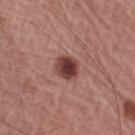Case summary:
* notes · total-body-photography surveillance lesion; no biopsy
* illumination · white-light illumination
* anatomic site · the left upper arm
* TBP lesion metrics · an area of roughly 6.5 mm², an eccentricity of roughly 0.3, and two-axis asymmetry of about 0.15; a mean CIELAB color near L≈41 a*≈23 b*≈22, roughly 15 lightness units darker than nearby skin, and a normalized lesion–skin contrast near 11.5; a border-irregularity rating of about 1.5/10 and a peripheral color-asymmetry measure near 1.5
* patient · male, about 65 years old
* image source · total-body-photography crop, ~15 mm field of view